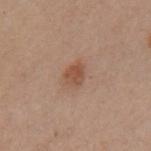{"biopsy_status": "not biopsied; imaged during a skin examination", "lighting": "cross-polarized", "image": {"source": "total-body photography crop", "field_of_view_mm": 15}, "site": "left upper arm", "patient": {"sex": "female", "age_approx": 30}, "lesion_size": {"long_diameter_mm_approx": 3.0}}